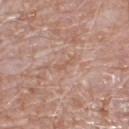The lesion was tiled from a total-body skin photograph and was not biopsied. Automated image analysis of the tile measured border irregularity of about 6.5 on a 0–10 scale, internal color variation of about 0 on a 0–10 scale, and radial color variation of about 0. And it measured an automated nevus-likeness rating near 0 out of 100 and a detector confidence of about 90 out of 100 that the crop contains a lesion. Imaged with white-light lighting. A 15 mm crop from a total-body photograph taken for skin-cancer surveillance. The recorded lesion diameter is about 2.5 mm. The subject is a male roughly 75 years of age. On the left lower leg.